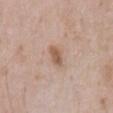Impression:
The lesion was photographed on a routine skin check and not biopsied; there is no pathology result.
Context:
A close-up tile cropped from a whole-body skin photograph, about 15 mm across. On the abdomen. Approximately 2.5 mm at its widest. Imaged with white-light lighting. A male subject, aged 58–62.